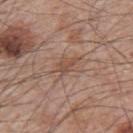No biopsy was performed on this lesion — it was imaged during a full skin examination and was not determined to be concerning. From the left upper arm. A male patient aged around 65. A region of skin cropped from a whole-body photographic capture, roughly 15 mm wide.